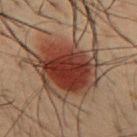Clinical impression:
The lesion was photographed on a routine skin check and not biopsied; there is no pathology result.